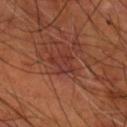| key | value |
|---|---|
| follow-up | no biopsy performed (imaged during a skin exam) |
| image | 15 mm crop, total-body photography |
| diameter | ~3 mm (longest diameter) |
| lighting | cross-polarized illumination |
| site | the right forearm |
| patient | male, aged approximately 55 |
| automated metrics | a footprint of about 5 mm², an eccentricity of roughly 0.65, and two-axis asymmetry of about 0.4; a lesion color around L≈32 a*≈24 b*≈25 in CIELAB and a normalized lesion–skin contrast near 6 |